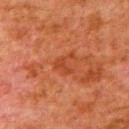notes=no biopsy performed (imaged during a skin exam) | body site=the left upper arm | patient=male, aged 78 to 82 | imaging modality=total-body-photography crop, ~15 mm field of view.